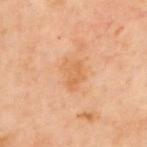{
  "biopsy_status": "not biopsied; imaged during a skin examination",
  "automated_metrics": {
    "color_variation_0_10": 2.5,
    "peripheral_color_asymmetry": 1.0,
    "nevus_likeness_0_100": 0
  },
  "image": {
    "source": "total-body photography crop",
    "field_of_view_mm": 15
  },
  "lesion_size": {
    "long_diameter_mm_approx": 3.5
  },
  "lighting": "cross-polarized",
  "patient": {
    "sex": "male",
    "age_approx": 65
  },
  "site": "upper back"
}